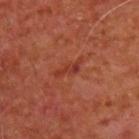The lesion was tiled from a total-body skin photograph and was not biopsied.
Approximately 3.5 mm at its widest.
Cropped from a whole-body photographic skin survey; the tile spans about 15 mm.
On the upper back.
A male subject, aged around 65.